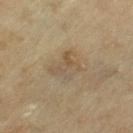Clinical impression:
Captured during whole-body skin photography for melanoma surveillance; the lesion was not biopsied.
Image and clinical context:
The lesion-visualizer software estimated an area of roughly 8 mm², a shape eccentricity near 0.55, and a symmetry-axis asymmetry near 0.4. The software also gave a nevus-likeness score of about 5/100 and a detector confidence of about 90 out of 100 that the crop contains a lesion. A region of skin cropped from a whole-body photographic capture, roughly 15 mm wide. A female patient, in their mid-50s. This is a cross-polarized tile. Approximately 3.5 mm at its widest. On the left thigh.